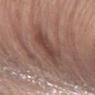{"biopsy_status": "not biopsied; imaged during a skin examination"}